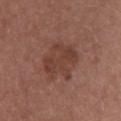This lesion was catalogued during total-body skin photography and was not selected for biopsy. A roughly 15 mm field-of-view crop from a total-body skin photograph. A female subject, about 60 years old. Located on the upper back. The lesion's longest dimension is about 5 mm.